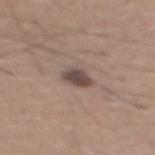biopsy status: total-body-photography surveillance lesion; no biopsy
body site: the mid back
imaging modality: ~15 mm tile from a whole-body skin photo
tile lighting: white-light illumination
patient: male, roughly 45 years of age
lesion size: ≈2.5 mm